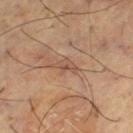Assessment: Part of a total-body skin-imaging series; this lesion was reviewed on a skin check and was not flagged for biopsy. Acquisition and patient details: Located on the left thigh. This image is a 15 mm lesion crop taken from a total-body photograph. The total-body-photography lesion software estimated an area of roughly 3.5 mm², an outline eccentricity of about 0.85 (0 = round, 1 = elongated), and a symmetry-axis asymmetry near 0.35. The analysis additionally found a border-irregularity index near 4/10, a color-variation rating of about 2/10, and peripheral color asymmetry of about 0.5. A male subject, aged approximately 65. The tile uses cross-polarized illumination. The lesion's longest dimension is about 3 mm.